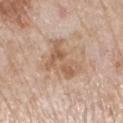lighting: white-light
automated_metrics:
  area_mm2_approx: 11.0
  eccentricity: 0.8
  shape_asymmetry: 0.55
lesion_size:
  long_diameter_mm_approx: 5.0
patient:
  sex: female
  age_approx: 70
image:
  source: total-body photography crop
  field_of_view_mm: 15
site: left lower leg